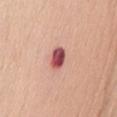{"biopsy_status": "not biopsied; imaged during a skin examination", "image": {"source": "total-body photography crop", "field_of_view_mm": 15}, "lighting": "white-light", "patient": {"sex": "female", "age_approx": 55}, "site": "front of the torso"}